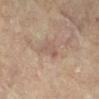{"biopsy_status": "not biopsied; imaged during a skin examination", "lesion_size": {"long_diameter_mm_approx": 3.5}, "image": {"source": "total-body photography crop", "field_of_view_mm": 15}, "lighting": "cross-polarized", "site": "leg", "patient": {"sex": "female", "age_approx": 80}}